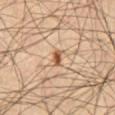No biopsy was performed on this lesion — it was imaged during a full skin examination and was not determined to be concerning.
A male subject, roughly 55 years of age.
Captured under cross-polarized illumination.
Measured at roughly 2 mm in maximum diameter.
A lesion tile, about 15 mm wide, cut from a 3D total-body photograph.
The lesion is on the front of the torso.